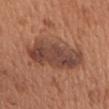- biopsy status · imaged on a skin check; not biopsied
- lesion diameter · ~7 mm (longest diameter)
- tile lighting · white-light
- patient · male, aged 63 to 67
- imaging modality · 15 mm crop, total-body photography
- site · the chest